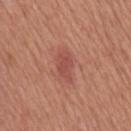Impression:
No biopsy was performed on this lesion — it was imaged during a full skin examination and was not determined to be concerning.
Clinical summary:
The subject is a male aged around 65. A roughly 15 mm field-of-view crop from a total-body skin photograph. On the chest. The total-body-photography lesion software estimated an area of roughly 5.5 mm², a shape eccentricity near 0.85, and a shape-asymmetry score of about 0.25 (0 = symmetric). The software also gave a lesion color around L≈50 a*≈28 b*≈27 in CIELAB, roughly 8 lightness units darker than nearby skin, and a lesion-to-skin contrast of about 6 (normalized; higher = more distinct).